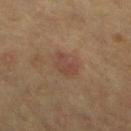biopsy status: no biopsy performed (imaged during a skin exam) | tile lighting: cross-polarized illumination | lesion diameter: about 3.5 mm | patient: female, aged around 55 | TBP lesion metrics: border irregularity of about 3.5 on a 0–10 scale, internal color variation of about 2.5 on a 0–10 scale, and a peripheral color-asymmetry measure near 1 | anatomic site: the left lower leg | image source: ~15 mm crop, total-body skin-cancer survey.